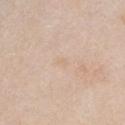notes = imaged on a skin check; not biopsied
subject = female, aged 38 to 42
lesion diameter = ~1.5 mm (longest diameter)
anatomic site = the chest
TBP lesion metrics = a shape eccentricity near 0.7 and a symmetry-axis asymmetry near 0.45; a mean CIELAB color near L≈70 a*≈15 b*≈30, a lesion–skin lightness drop of about 4, and a normalized border contrast of about 3.5; lesion-presence confidence of about 100/100
image = ~15 mm crop, total-body skin-cancer survey
illumination = white-light